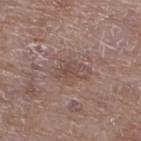Q: Is there a histopathology result?
A: catalogued during a skin exam; not biopsied
Q: Automated lesion metrics?
A: a lesion area of about 5.5 mm², a shape eccentricity near 0.85, and a symmetry-axis asymmetry near 0.35; a lesion color around L≈48 a*≈17 b*≈21 in CIELAB, about 7 CIELAB-L* units darker than the surrounding skin, and a normalized lesion–skin contrast near 5.5; radial color variation of about 0.5; an automated nevus-likeness rating near 0 out of 100 and a lesion-detection confidence of about 100/100
Q: How large is the lesion?
A: about 4 mm
Q: What lighting was used for the tile?
A: white-light
Q: How was this image acquired?
A: ~15 mm crop, total-body skin-cancer survey
Q: Where on the body is the lesion?
A: the left lower leg
Q: Patient demographics?
A: female, roughly 85 years of age or older